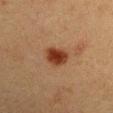Imaged during a routine full-body skin examination; the lesion was not biopsied and no histopathology is available. The lesion is on the arm. Measured at roughly 3 mm in maximum diameter. A close-up tile cropped from a whole-body skin photograph, about 15 mm across. Imaged with cross-polarized lighting. A male patient, in their 40s.